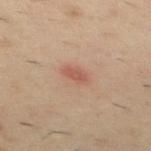<lesion>
  <biopsy_status>not biopsied; imaged during a skin examination</biopsy_status>
</lesion>